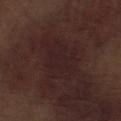This lesion was catalogued during total-body skin photography and was not selected for biopsy.
Automated image analysis of the tile measured a footprint of about 15 mm², an outline eccentricity of about 0.75 (0 = round, 1 = elongated), and two-axis asymmetry of about 0.3. The software also gave an automated nevus-likeness rating near 0 out of 100 and lesion-presence confidence of about 80/100.
Captured under white-light illumination.
The subject is a male aged around 70.
Measured at roughly 5.5 mm in maximum diameter.
Cropped from a whole-body photographic skin survey; the tile spans about 15 mm.
From the left lower leg.